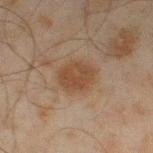The lesion was tiled from a total-body skin photograph and was not biopsied. This image is a 15 mm lesion crop taken from a total-body photograph. The subject is a male in their mid-40s. From the left thigh. An algorithmic analysis of the crop reported a footprint of about 9 mm² and an eccentricity of roughly 0.65. The software also gave a mean CIELAB color near L≈37 a*≈15 b*≈26, roughly 7 lightness units darker than nearby skin, and a normalized border contrast of about 7.5. It also reported a classifier nevus-likeness of about 65/100. Captured under cross-polarized illumination. The lesion's longest dimension is about 3.5 mm.